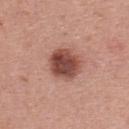No biopsy was performed on this lesion — it was imaged during a full skin examination and was not determined to be concerning. Longest diameter approximately 4 mm. The lesion is located on the back. Captured under white-light illumination. A female subject, aged around 40. Automated tile analysis of the lesion measured a lesion area of about 12 mm², a shape eccentricity near 0.45, and two-axis asymmetry of about 0.15. And it measured a border-irregularity index near 1/10, a within-lesion color-variation index near 5/10, and radial color variation of about 1.5. And it measured an automated nevus-likeness rating near 85 out of 100 and a lesion-detection confidence of about 100/100. A 15 mm crop from a total-body photograph taken for skin-cancer surveillance.